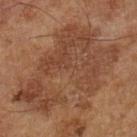The lesion was tiled from a total-body skin photograph and was not biopsied.
The patient is a male approximately 65 years of age.
A 15 mm close-up extracted from a 3D total-body photography capture.
The tile uses cross-polarized illumination.